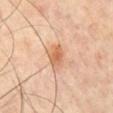Findings:
- biopsy status — total-body-photography surveillance lesion; no biopsy
- automated metrics — a footprint of about 5 mm², an eccentricity of roughly 0.7, and two-axis asymmetry of about 0.2; a lesion color around L≈65 a*≈24 b*≈37 in CIELAB, about 11 CIELAB-L* units darker than the surrounding skin, and a normalized lesion–skin contrast near 7.5; a border-irregularity rating of about 2/10, a color-variation rating of about 3.5/10, and radial color variation of about 1; an automated nevus-likeness rating near 60 out of 100 and a detector confidence of about 100 out of 100 that the crop contains a lesion
- illumination — cross-polarized
- subject — male, roughly 55 years of age
- diameter — ~2.5 mm (longest diameter)
- image — 15 mm crop, total-body photography
- location — the front of the torso